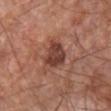{
  "biopsy_status": "not biopsied; imaged during a skin examination",
  "lighting": "white-light",
  "automated_metrics": {
    "area_mm2_approx": 8.5,
    "eccentricity": 0.5,
    "shape_asymmetry": 0.3,
    "vs_skin_darker_L": 12.0,
    "vs_skin_contrast_norm": 9.5,
    "nevus_likeness_0_100": 30,
    "lesion_detection_confidence_0_100": 100
  },
  "lesion_size": {
    "long_diameter_mm_approx": 3.5
  },
  "patient": {
    "sex": "male",
    "age_approx": 65
  },
  "image": {
    "source": "total-body photography crop",
    "field_of_view_mm": 15
  },
  "site": "front of the torso"
}